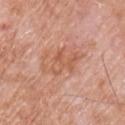Captured during whole-body skin photography for melanoma surveillance; the lesion was not biopsied.